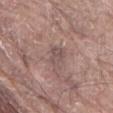biopsy status — catalogued during a skin exam; not biopsied
lesion size — ~3 mm (longest diameter)
tile lighting — white-light illumination
image source — ~15 mm tile from a whole-body skin photo
subject — male, aged 78 to 82
body site — the abdomen